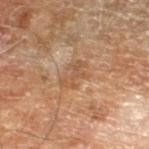subject: male, aged 68–72 | illumination: cross-polarized | image source: ~15 mm crop, total-body skin-cancer survey | TBP lesion metrics: a shape eccentricity near 0.8 and a shape-asymmetry score of about 0.35 (0 = symmetric); an average lesion color of about L≈53 a*≈20 b*≈33 (CIELAB) and a lesion-to-skin contrast of about 5 (normalized; higher = more distinct); a nevus-likeness score of about 0/100 | location: the left lower leg.